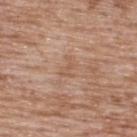{
  "biopsy_status": "not biopsied; imaged during a skin examination",
  "lesion_size": {
    "long_diameter_mm_approx": 2.5
  },
  "patient": {
    "sex": "male",
    "age_approx": 50
  },
  "site": "upper back",
  "image": {
    "source": "total-body photography crop",
    "field_of_view_mm": 15
  }
}